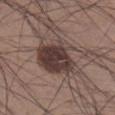Captured during whole-body skin photography for melanoma surveillance; the lesion was not biopsied. Located on the right lower leg. The recorded lesion diameter is about 6 mm. A 15 mm crop from a total-body photograph taken for skin-cancer surveillance. The tile uses white-light illumination. The subject is a male about 35 years old. Automated image analysis of the tile measured a border-irregularity index near 3.5/10, a within-lesion color-variation index near 6.5/10, and radial color variation of about 2.